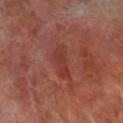Impression: Recorded during total-body skin imaging; not selected for excision or biopsy. Acquisition and patient details: A 15 mm close-up tile from a total-body photography series done for melanoma screening. Imaged with cross-polarized lighting. The subject is a male aged around 70. Located on the right lower leg. Measured at roughly 4 mm in maximum diameter.